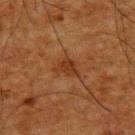This lesion was catalogued during total-body skin photography and was not selected for biopsy.
The subject is a male in their mid- to late 60s.
A lesion tile, about 15 mm wide, cut from a 3D total-body photograph.
From the upper back.
Measured at roughly 3 mm in maximum diameter.
The total-body-photography lesion software estimated an area of roughly 4.5 mm², an eccentricity of roughly 0.75, and two-axis asymmetry of about 0.4. And it measured a border-irregularity rating of about 4/10, a within-lesion color-variation index near 1.5/10, and radial color variation of about 0.5.
This is a cross-polarized tile.